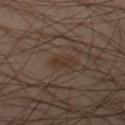Q: Is there a histopathology result?
A: catalogued during a skin exam; not biopsied
Q: What is the lesion's diameter?
A: ~3 mm (longest diameter)
Q: Where on the body is the lesion?
A: the left thigh
Q: Patient demographics?
A: male, aged around 50
Q: How was this image acquired?
A: ~15 mm tile from a whole-body skin photo
Q: How was the tile lit?
A: cross-polarized illumination